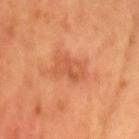notes — catalogued during a skin exam; not biopsied | image — ~15 mm crop, total-body skin-cancer survey | patient — male, in their mid- to late 50s | lighting — cross-polarized | location — the head or neck.